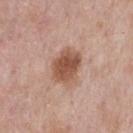notes=no biopsy performed (imaged during a skin exam); imaging modality=~15 mm crop, total-body skin-cancer survey; patient=male, aged around 55; location=the chest.